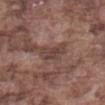{
  "automated_metrics": {
    "border_irregularity_0_10": 6.0,
    "color_variation_0_10": 2.5,
    "peripheral_color_asymmetry": 0.5,
    "nevus_likeness_0_100": 0,
    "lesion_detection_confidence_0_100": 50
  },
  "image": {
    "source": "total-body photography crop",
    "field_of_view_mm": 15
  },
  "lesion_size": {
    "long_diameter_mm_approx": 3.5
  },
  "lighting": "white-light",
  "patient": {
    "sex": "male",
    "age_approx": 75
  },
  "site": "abdomen"
}